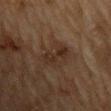{
  "biopsy_status": "not biopsied; imaged during a skin examination",
  "lighting": "cross-polarized",
  "image": {
    "source": "total-body photography crop",
    "field_of_view_mm": 15
  },
  "automated_metrics": {
    "area_mm2_approx": 5.0,
    "eccentricity": 0.85,
    "shape_asymmetry": 0.35,
    "nevus_likeness_0_100": 10,
    "lesion_detection_confidence_0_100": 100
  },
  "site": "back",
  "patient": {
    "sex": "male",
    "age_approx": "85+"
  }
}